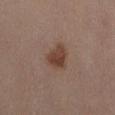Background: The subject is a female approximately 30 years of age. Automated tile analysis of the lesion measured a classifier nevus-likeness of about 95/100 and lesion-presence confidence of about 100/100. Located on the abdomen. This image is a 15 mm lesion crop taken from a total-body photograph. The tile uses white-light illumination.